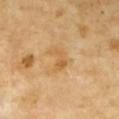automated lesion analysis: a footprint of about 4 mm², an eccentricity of roughly 0.8, and two-axis asymmetry of about 0.45; a mean CIELAB color near L≈60 a*≈20 b*≈43, a lesion–skin lightness drop of about 8, and a normalized border contrast of about 5.5; an automated nevus-likeness rating near 0 out of 100 and lesion-presence confidence of about 100/100
lesion size: ≈3 mm
anatomic site: the chest
imaging modality: 15 mm crop, total-body photography
patient: female, approximately 55 years of age